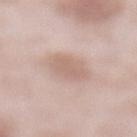Imaged during a routine full-body skin examination; the lesion was not biopsied and no histopathology is available.
A male subject aged 53–57.
A 15 mm close-up tile from a total-body photography series done for melanoma screening.
The lesion is located on the front of the torso.